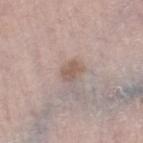Captured during whole-body skin photography for melanoma surveillance; the lesion was not biopsied. A male subject about 80 years old. A close-up tile cropped from a whole-body skin photograph, about 15 mm across. The lesion is on the right thigh. The recorded lesion diameter is about 2.5 mm.